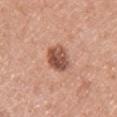Impression: Imaged during a routine full-body skin examination; the lesion was not biopsied and no histopathology is available. Image and clinical context: A roughly 15 mm field-of-view crop from a total-body skin photograph. On the left upper arm. The lesion-visualizer software estimated an average lesion color of about L≈52 a*≈24 b*≈29 (CIELAB), a lesion–skin lightness drop of about 15, and a normalized border contrast of about 10. The analysis additionally found a border-irregularity index near 1.5/10, internal color variation of about 5.5 on a 0–10 scale, and peripheral color asymmetry of about 2. The patient is a female approximately 50 years of age. Captured under white-light illumination. The lesion's longest dimension is about 3.5 mm.